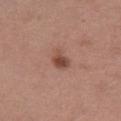{"biopsy_status": "not biopsied; imaged during a skin examination", "lesion_size": {"long_diameter_mm_approx": 2.5}, "image": {"source": "total-body photography crop", "field_of_view_mm": 15}, "lighting": "white-light", "site": "left thigh", "patient": {"sex": "female", "age_approx": 50}}